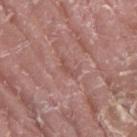Findings:
– follow-up · imaged on a skin check; not biopsied
– image · ~15 mm crop, total-body skin-cancer survey
– location · the right thigh
– subject · male, roughly 40 years of age